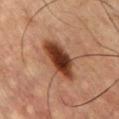Q: Lesion location?
A: the chest
Q: Automated lesion metrics?
A: an area of roughly 13 mm², a shape eccentricity near 0.9, and a shape-asymmetry score of about 0.2 (0 = symmetric); an average lesion color of about L≈39 a*≈24 b*≈31 (CIELAB), a lesion–skin lightness drop of about 18, and a normalized lesion–skin contrast near 13.5; border irregularity of about 2.5 on a 0–10 scale, a within-lesion color-variation index near 6.5/10, and a peripheral color-asymmetry measure near 1.5; a nevus-likeness score of about 50/100
Q: How was the tile lit?
A: cross-polarized
Q: How was this image acquired?
A: ~15 mm tile from a whole-body skin photo
Q: Lesion size?
A: ~6 mm (longest diameter)
Q: Who is the patient?
A: male, in their mid-50s